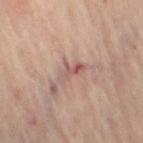Captured under cross-polarized illumination.
A female patient, aged 58–62.
From the right thigh.
A 15 mm crop from a total-body photograph taken for skin-cancer surveillance.
Longest diameter approximately 3.5 mm.
Automated tile analysis of the lesion measured a mean CIELAB color near L≈55 a*≈22 b*≈22, a lesion–skin lightness drop of about 8, and a normalized border contrast of about 6.